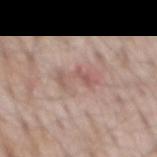  patient:
    sex: male
    age_approx: 65
  image:
    source: total-body photography crop
    field_of_view_mm: 15
  automated_metrics:
    area_mm2_approx: 6.5
    eccentricity: 0.9
    shape_asymmetry: 0.5
    border_irregularity_0_10: 7.5
    color_variation_0_10: 2.5
    peripheral_color_asymmetry: 0.5
  site: back